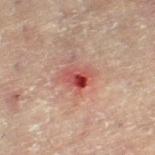  image:
    source: total-body photography crop
    field_of_view_mm: 15
  lighting: cross-polarized
  automated_metrics:
    border_irregularity_0_10: 3.0
    color_variation_0_10: 10.0
    nevus_likeness_0_100: 0
  patient:
    sex: female
    age_approx: 80
  site: right leg